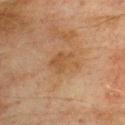• lesion size · about 4 mm
• image · ~15 mm tile from a whole-body skin photo
• subject · male, about 75 years old
• tile lighting · cross-polarized illumination
• anatomic site · the upper back